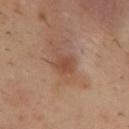Located on the upper back. The subject is a male approximately 40 years of age. A 15 mm close-up extracted from a 3D total-body photography capture.